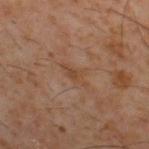<tbp_lesion>
  <biopsy_status>not biopsied; imaged during a skin examination</biopsy_status>
  <site>upper back</site>
  <patient>
    <sex>male</sex>
    <age_approx>60</age_approx>
  </patient>
  <image>
    <source>total-body photography crop</source>
    <field_of_view_mm>15</field_of_view_mm>
  </image>
</tbp_lesion>